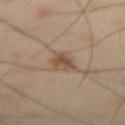follow-up=catalogued during a skin exam; not biopsied | body site=the abdomen | image=~15 mm crop, total-body skin-cancer survey | subject=male, approximately 55 years of age.